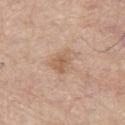Q: Is there a histopathology result?
A: imaged on a skin check; not biopsied
Q: What are the patient's age and sex?
A: male, roughly 70 years of age
Q: What is the anatomic site?
A: the leg
Q: What is the imaging modality?
A: total-body-photography crop, ~15 mm field of view
Q: Illumination type?
A: white-light illumination
Q: Automated lesion metrics?
A: a lesion area of about 10 mm²; a border-irregularity rating of about 4.5/10, a within-lesion color-variation index near 4/10, and a peripheral color-asymmetry measure near 1.5; a classifier nevus-likeness of about 0/100 and lesion-presence confidence of about 100/100
Q: How large is the lesion?
A: ~4 mm (longest diameter)